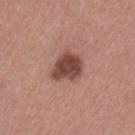Impression:
Imaged during a routine full-body skin examination; the lesion was not biopsied and no histopathology is available.
Background:
From the left thigh. The recorded lesion diameter is about 4 mm. A female patient aged around 60. This image is a 15 mm lesion crop taken from a total-body photograph. Automated tile analysis of the lesion measured internal color variation of about 3.5 on a 0–10 scale and peripheral color asymmetry of about 1.